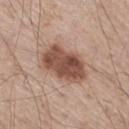  biopsy_status: not biopsied; imaged during a skin examination
  patient:
    sex: male
    age_approx: 60
  image:
    source: total-body photography crop
    field_of_view_mm: 15
  site: right thigh